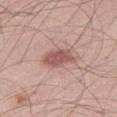No biopsy was performed on this lesion — it was imaged during a full skin examination and was not determined to be concerning.
Imaged with white-light lighting.
The lesion is on the right thigh.
The lesion's longest dimension is about 4 mm.
A male subject approximately 55 years of age.
A close-up tile cropped from a whole-body skin photograph, about 15 mm across.